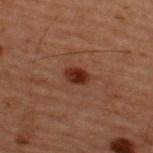{
  "patient": {
    "sex": "male",
    "age_approx": 60
  },
  "image": {
    "source": "total-body photography crop",
    "field_of_view_mm": 15
  },
  "automated_metrics": {
    "nevus_likeness_0_100": 95,
    "lesion_detection_confidence_0_100": 100
  },
  "site": "upper back"
}